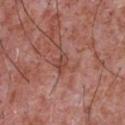notes — total-body-photography surveillance lesion; no biopsy | image source — total-body-photography crop, ~15 mm field of view | tile lighting — white-light | lesion size — ~2.5 mm (longest diameter) | subject — male, roughly 45 years of age | site — the chest.